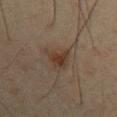<record>
<biopsy_status>not biopsied; imaged during a skin examination</biopsy_status>
<automated_metrics>
  <border_irregularity_0_10>5.5</border_irregularity_0_10>
  <color_variation_0_10>3.0</color_variation_0_10>
</automated_metrics>
<patient>
  <sex>male</sex>
  <age_approx>35</age_approx>
</patient>
<lesion_size>
  <long_diameter_mm_approx>3.5</long_diameter_mm_approx>
</lesion_size>
<site>left upper arm</site>
<image>
  <source>total-body photography crop</source>
  <field_of_view_mm>15</field_of_view_mm>
</image>
</record>